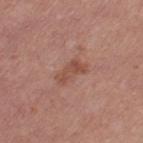* biopsy status · catalogued during a skin exam; not biopsied
* acquisition · total-body-photography crop, ~15 mm field of view
* subject · female, aged 28 to 32
* tile lighting · white-light illumination
* automated metrics · a lesion area of about 5 mm², an outline eccentricity of about 0.9 (0 = round, 1 = elongated), and two-axis asymmetry of about 0.4; an average lesion color of about L≈49 a*≈23 b*≈28 (CIELAB) and a lesion-to-skin contrast of about 6.5 (normalized; higher = more distinct); a border-irregularity index near 4.5/10, internal color variation of about 2.5 on a 0–10 scale, and peripheral color asymmetry of about 0.5
* anatomic site · the left thigh
* size · ~3.5 mm (longest diameter)